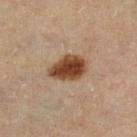  image:
    source: total-body photography crop
    field_of_view_mm: 15
  site: left lower leg
  lighting: cross-polarized
  lesion_size:
    long_diameter_mm_approx: 4.5
  patient:
    sex: female
    age_approx: 60